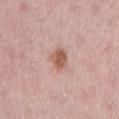| key | value |
|---|---|
| follow-up | imaged on a skin check; not biopsied |
| location | the lower back |
| acquisition | total-body-photography crop, ~15 mm field of view |
| TBP lesion metrics | two-axis asymmetry of about 0.3; a border-irregularity index near 2.5/10, a color-variation rating of about 3/10, and a peripheral color-asymmetry measure near 1 |
| subject | female, approximately 65 years of age |